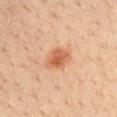<case>
  <biopsy_status>not biopsied; imaged during a skin examination</biopsy_status>
  <site>left upper arm</site>
  <lighting>cross-polarized</lighting>
  <lesion_size>
    <long_diameter_mm_approx>3.5</long_diameter_mm_approx>
  </lesion_size>
  <automated_metrics>
    <area_mm2_approx>7.0</area_mm2_approx>
    <eccentricity>0.7</eccentricity>
    <shape_asymmetry>0.25</shape_asymmetry>
    <cielab_L>55</cielab_L>
    <cielab_a>23</cielab_a>
    <cielab_b>35</cielab_b>
    <vs_skin_darker_L>11.0</vs_skin_darker_L>
    <vs_skin_contrast_norm>7.5</vs_skin_contrast_norm>
  </automated_metrics>
  <patient>
    <sex>male</sex>
    <age_approx>35</age_approx>
  </patient>
  <image>
    <source>total-body photography crop</source>
    <field_of_view_mm>15</field_of_view_mm>
  </image>
</case>